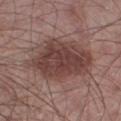Captured during whole-body skin photography for melanoma surveillance; the lesion was not biopsied.